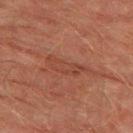A roughly 15 mm field-of-view crop from a total-body skin photograph. The tile uses cross-polarized illumination. A male subject, aged 73 to 77. Approximately 5 mm at its widest. The total-body-photography lesion software estimated a footprint of about 6 mm² and an outline eccentricity of about 0.95 (0 = round, 1 = elongated). The lesion is on the left thigh.